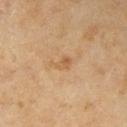Clinical impression:
Imaged during a routine full-body skin examination; the lesion was not biopsied and no histopathology is available.
Image and clinical context:
A female subject approximately 60 years of age. This is a cross-polarized tile. On the right upper arm. About 2.5 mm across. A close-up tile cropped from a whole-body skin photograph, about 15 mm across.